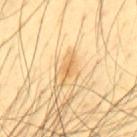| key | value |
|---|---|
| biopsy status | total-body-photography surveillance lesion; no biopsy |
| patient | male, approximately 45 years of age |
| size | ≈2 mm |
| tile lighting | cross-polarized illumination |
| imaging modality | ~15 mm tile from a whole-body skin photo |
| location | the back |